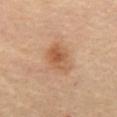This lesion was catalogued during total-body skin photography and was not selected for biopsy. A female subject, approximately 65 years of age. The lesion is located on the abdomen. Cropped from a total-body skin-imaging series; the visible field is about 15 mm.